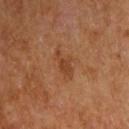workup: imaged on a skin check; not biopsied
site: the right upper arm
subject: male, in their mid-60s
diameter: ~3.5 mm (longest diameter)
illumination: cross-polarized
TBP lesion metrics: an area of roughly 4.5 mm², a shape eccentricity near 0.85, and a shape-asymmetry score of about 0.25 (0 = symmetric); a lesion–skin lightness drop of about 7
acquisition: ~15 mm tile from a whole-body skin photo